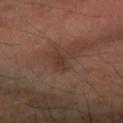The lesion was tiled from a total-body skin photograph and was not biopsied.
The lesion-visualizer software estimated a footprint of about 4.5 mm² and a shape eccentricity near 0.7. And it measured a classifier nevus-likeness of about 0/100 and a detector confidence of about 100 out of 100 that the crop contains a lesion.
From the right forearm.
The tile uses cross-polarized illumination.
A male patient in their mid- to late 40s.
A region of skin cropped from a whole-body photographic capture, roughly 15 mm wide.
About 3 mm across.